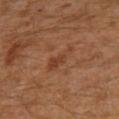The lesion was tiled from a total-body skin photograph and was not biopsied. A lesion tile, about 15 mm wide, cut from a 3D total-body photograph. The subject is a male aged around 30. On the left leg. Approximately 4.5 mm at its widest.